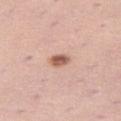notes: no biopsy performed (imaged during a skin exam) | image: total-body-photography crop, ~15 mm field of view | subject: female, about 40 years old | illumination: white-light illumination | location: the left leg | automated metrics: a lesion area of about 3.5 mm² and an outline eccentricity of about 0.8 (0 = round, 1 = elongated); a mean CIELAB color near L≈58 a*≈23 b*≈28, a lesion–skin lightness drop of about 15, and a lesion-to-skin contrast of about 9 (normalized; higher = more distinct); a border-irregularity index near 1/10, a within-lesion color-variation index near 4/10, and radial color variation of about 1.5; an automated nevus-likeness rating near 95 out of 100 and lesion-presence confidence of about 100/100.